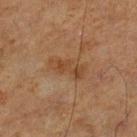Recorded during total-body skin imaging; not selected for excision or biopsy. The lesion is on the right lower leg. This image is a 15 mm lesion crop taken from a total-body photograph. A male patient, in their mid-60s.